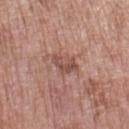biopsy status: imaged on a skin check; not biopsied | anatomic site: the left upper arm | automated metrics: a shape eccentricity near 0.7 and two-axis asymmetry of about 0.25; an average lesion color of about L≈50 a*≈22 b*≈25 (CIELAB), about 9 CIELAB-L* units darker than the surrounding skin, and a normalized lesion–skin contrast near 6.5; border irregularity of about 3 on a 0–10 scale, internal color variation of about 4 on a 0–10 scale, and radial color variation of about 1.5 | patient: female, aged 68–72 | lighting: white-light illumination | image: ~15 mm tile from a whole-body skin photo | lesion diameter: about 3 mm.